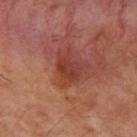notes: no biopsy performed (imaged during a skin exam) | lighting: cross-polarized illumination | lesion size: about 3.5 mm | imaging modality: total-body-photography crop, ~15 mm field of view | site: the right upper arm | image-analysis metrics: a lesion area of about 6 mm²; a lesion color around L≈40 a*≈30 b*≈28 in CIELAB, roughly 8 lightness units darker than nearby skin, and a lesion-to-skin contrast of about 7 (normalized; higher = more distinct) | patient: male, aged 53 to 57.